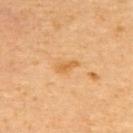Part of a total-body skin-imaging series; this lesion was reviewed on a skin check and was not flagged for biopsy.
A 15 mm close-up tile from a total-body photography series done for melanoma screening.
Located on the upper back.
This is a cross-polarized tile.
The recorded lesion diameter is about 2.5 mm.
The total-body-photography lesion software estimated an area of roughly 3 mm², an outline eccentricity of about 0.9 (0 = round, 1 = elongated), and a symmetry-axis asymmetry near 0.3. The analysis additionally found an average lesion color of about L≈62 a*≈23 b*≈46 (CIELAB) and about 8 CIELAB-L* units darker than the surrounding skin.
A male patient, aged approximately 60.